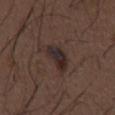notes = imaged on a skin check; not biopsied | subject = male, aged approximately 50 | size = ≈4 mm | body site = the abdomen | lighting = white-light illumination | image source = total-body-photography crop, ~15 mm field of view | automated lesion analysis = radial color variation of about 2.5.